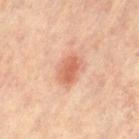follow-up: catalogued during a skin exam; not biopsied
subject: female, roughly 65 years of age
location: the leg
lighting: cross-polarized illumination
TBP lesion metrics: an area of roughly 7 mm², an outline eccentricity of about 0.75 (0 = round, 1 = elongated), and a symmetry-axis asymmetry near 0.25; border irregularity of about 2.5 on a 0–10 scale, internal color variation of about 3 on a 0–10 scale, and radial color variation of about 1
image: ~15 mm tile from a whole-body skin photo
lesion size: ~3.5 mm (longest diameter)